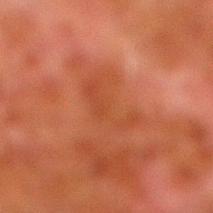| field | value |
|---|---|
| follow-up | no biopsy performed (imaged during a skin exam) |
| acquisition | 15 mm crop, total-body photography |
| lesion size | ~5 mm (longest diameter) |
| anatomic site | the right lower leg |
| subject | male, aged 78–82 |
| lighting | cross-polarized illumination |